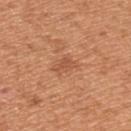Assessment: Captured during whole-body skin photography for melanoma surveillance; the lesion was not biopsied. Image and clinical context: From the arm. The tile uses white-light illumination. A male patient, approximately 65 years of age. Longest diameter approximately 3 mm. A region of skin cropped from a whole-body photographic capture, roughly 15 mm wide.